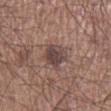This lesion was catalogued during total-body skin photography and was not selected for biopsy. Cropped from a total-body skin-imaging series; the visible field is about 15 mm. Located on the right lower leg. A male subject, aged approximately 60.